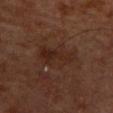Recorded during total-body skin imaging; not selected for excision or biopsy. The tile uses cross-polarized illumination. The lesion-visualizer software estimated a footprint of about 12 mm², an eccentricity of roughly 0.85, and two-axis asymmetry of about 0.25. It also reported a normalized lesion–skin contrast near 6. And it measured a border-irregularity index near 3.5/10, internal color variation of about 4 on a 0–10 scale, and peripheral color asymmetry of about 1.5. A region of skin cropped from a whole-body photographic capture, roughly 15 mm wide. A male patient roughly 60 years of age. Located on the upper back.